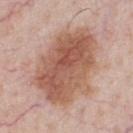| feature | finding |
|---|---|
| follow-up | total-body-photography surveillance lesion; no biopsy |
| anatomic site | the chest |
| subject | male, in their 60s |
| tile lighting | white-light |
| automated lesion analysis | a footprint of about 47 mm², an eccentricity of roughly 0.75, and a symmetry-axis asymmetry near 0.15; border irregularity of about 2.5 on a 0–10 scale and a color-variation rating of about 6.5/10 |
| diameter | about 9.5 mm |
| acquisition | ~15 mm crop, total-body skin-cancer survey |